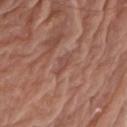Findings:
* workup · no biopsy performed (imaged during a skin exam)
* subject · male, aged approximately 80
* body site · the arm
* acquisition · 15 mm crop, total-body photography